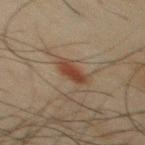• biopsy status — total-body-photography surveillance lesion; no biopsy
• TBP lesion metrics — a lesion–skin lightness drop of about 9 and a normalized border contrast of about 9; a within-lesion color-variation index near 2.5/10 and radial color variation of about 1
• anatomic site — the chest
• subject — male, approximately 45 years of age
• lesion diameter — ≈4 mm
• imaging modality — ~15 mm tile from a whole-body skin photo